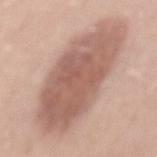Image and clinical context: A female subject, aged 33 to 37. From the front of the torso. Cropped from a total-body skin-imaging series; the visible field is about 15 mm. The lesion's longest dimension is about 13.5 mm. The tile uses white-light illumination.